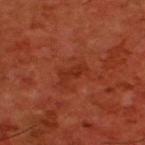{
  "biopsy_status": "not biopsied; imaged during a skin examination",
  "lighting": "cross-polarized",
  "site": "back",
  "image": {
    "source": "total-body photography crop",
    "field_of_view_mm": 15
  },
  "lesion_size": {
    "long_diameter_mm_approx": 3.0
  },
  "automated_metrics": {
    "cielab_L": 30,
    "cielab_a": 30,
    "cielab_b": 33,
    "vs_skin_darker_L": 6.0,
    "vs_skin_contrast_norm": 6.0
  },
  "patient": {
    "sex": "male",
    "age_approx": 60
  }
}